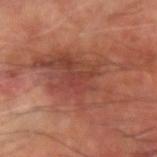This lesion was catalogued during total-body skin photography and was not selected for biopsy.
A region of skin cropped from a whole-body photographic capture, roughly 15 mm wide.
The recorded lesion diameter is about 10.5 mm.
The subject is a male approximately 60 years of age.
The lesion is located on the right arm.